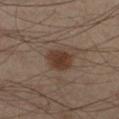Case summary:
* workup · catalogued during a skin exam; not biopsied
* lighting · cross-polarized illumination
* patient · male, about 55 years old
* size · ≈3 mm
* site · the left lower leg
* imaging modality · 15 mm crop, total-body photography
* TBP lesion metrics · internal color variation of about 2.5 on a 0–10 scale and radial color variation of about 1